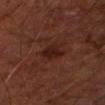image:
  source: total-body photography crop
  field_of_view_mm: 15
lesion_size:
  long_diameter_mm_approx: 3.0
lighting: cross-polarized
patient:
  sex: male
  age_approx: 65
site: arm